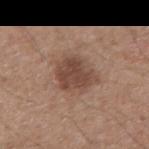notes: total-body-photography surveillance lesion; no biopsy | image source: 15 mm crop, total-body photography | body site: the right upper arm | patient: male, roughly 65 years of age | automated lesion analysis: a footprint of about 12 mm² and a shape-asymmetry score of about 0.25 (0 = symmetric); an average lesion color of about L≈45 a*≈19 b*≈26 (CIELAB), about 11 CIELAB-L* units darker than the surrounding skin, and a lesion-to-skin contrast of about 8 (normalized; higher = more distinct); border irregularity of about 2.5 on a 0–10 scale and a color-variation rating of about 2.5/10; a classifier nevus-likeness of about 45/100 and lesion-presence confidence of about 100/100 | diameter: ≈4.5 mm | illumination: white-light.